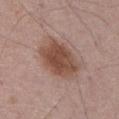Impression:
Imaged during a routine full-body skin examination; the lesion was not biopsied and no histopathology is available.
Background:
Automated image analysis of the tile measured a lesion area of about 18 mm², a shape eccentricity near 0.7, and a symmetry-axis asymmetry near 0.1. It also reported an average lesion color of about L≈48 a*≈20 b*≈26 (CIELAB) and a lesion–skin lightness drop of about 12. The software also gave a border-irregularity index near 1.5/10, a within-lesion color-variation index near 4/10, and radial color variation of about 1.5. Longest diameter approximately 5.5 mm. A close-up tile cropped from a whole-body skin photograph, about 15 mm across. On the abdomen. A male patient, aged 53–57. This is a white-light tile.